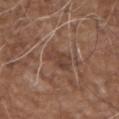Imaged during a routine full-body skin examination; the lesion was not biopsied and no histopathology is available. The lesion-visualizer software estimated an area of roughly 6 mm², an eccentricity of roughly 0.65, and a symmetry-axis asymmetry near 0.3. And it measured a border-irregularity index near 3/10, internal color variation of about 3.5 on a 0–10 scale, and a peripheral color-asymmetry measure near 1.5. Cropped from a total-body skin-imaging series; the visible field is about 15 mm. Imaged with white-light lighting. The patient is a male aged 73–77. Measured at roughly 3 mm in maximum diameter. On the left upper arm.